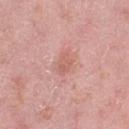Captured during whole-body skin photography for melanoma surveillance; the lesion was not biopsied.
From the leg.
A 15 mm close-up extracted from a 3D total-body photography capture.
Longest diameter approximately 2.5 mm.
A female patient aged approximately 40.